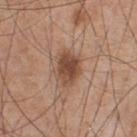biopsy status: imaged on a skin check; not biopsied.